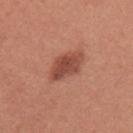workup = no biopsy performed (imaged during a skin exam) | imaging modality = 15 mm crop, total-body photography | illumination = white-light illumination | body site = the left upper arm | patient = female, about 40 years old.